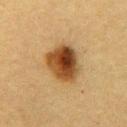notes: imaged on a skin check; not biopsied | anatomic site: the chest | patient: female, aged around 55 | diameter: ≈5 mm | acquisition: 15 mm crop, total-body photography.